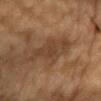This lesion was catalogued during total-body skin photography and was not selected for biopsy.
A 15 mm close-up tile from a total-body photography series done for melanoma screening.
Captured under cross-polarized illumination.
From the chest.
A male patient, aged 83 or older.